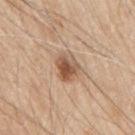No biopsy was performed on this lesion — it was imaged during a full skin examination and was not determined to be concerning.
Imaged with white-light lighting.
A roughly 15 mm field-of-view crop from a total-body skin photograph.
From the mid back.
The lesion's longest dimension is about 3.5 mm.
A male patient, aged around 80.